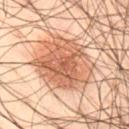Findings:
* biopsy status · catalogued during a skin exam; not biopsied
* subject · male, in their 50s
* imaging modality · 15 mm crop, total-body photography
* site · the left thigh
* tile lighting · cross-polarized illumination
* TBP lesion metrics · a lesion area of about 33 mm², a shape eccentricity near 0.55, and two-axis asymmetry of about 0.15; a mean CIELAB color near L≈46 a*≈19 b*≈27 and about 11 CIELAB-L* units darker than the surrounding skin; border irregularity of about 2 on a 0–10 scale and radial color variation of about 1.5; a detector confidence of about 100 out of 100 that the crop contains a lesion